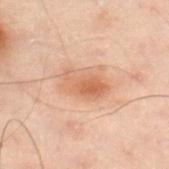No biopsy was performed on this lesion — it was imaged during a full skin examination and was not determined to be concerning.